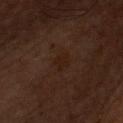Clinical summary:
From the chest. This image is a 15 mm lesion crop taken from a total-body photograph. Imaged with cross-polarized lighting. Automated tile analysis of the lesion measured a lesion area of about 4 mm², an outline eccentricity of about 0.75 (0 = round, 1 = elongated), and two-axis asymmetry of about 0.25. And it measured a border-irregularity index near 2.5/10, a color-variation rating of about 1/10, and peripheral color asymmetry of about 0.5. The software also gave a nevus-likeness score of about 0/100 and a lesion-detection confidence of about 100/100. The recorded lesion diameter is about 2.5 mm. A male subject in their 60s.